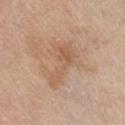Part of a total-body skin-imaging series; this lesion was reviewed on a skin check and was not flagged for biopsy. A 15 mm crop from a total-body photograph taken for skin-cancer surveillance. A female subject, roughly 65 years of age. Located on the chest.